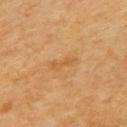Clinical impression:
Part of a total-body skin-imaging series; this lesion was reviewed on a skin check and was not flagged for biopsy.
Acquisition and patient details:
Approximately 3 mm at its widest. The total-body-photography lesion software estimated an average lesion color of about L≈54 a*≈21 b*≈40 (CIELAB). And it measured an automated nevus-likeness rating near 0 out of 100 and a lesion-detection confidence of about 100/100. The subject is a male in their 60s. A region of skin cropped from a whole-body photographic capture, roughly 15 mm wide. This is a cross-polarized tile. On the left upper arm.